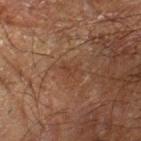Findings:
– workup · catalogued during a skin exam; not biopsied
– image · total-body-photography crop, ~15 mm field of view
– location · the arm
– lesion diameter · about 2.5 mm
– illumination · cross-polarized
– patient · male, aged approximately 65